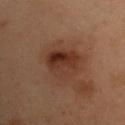site: the right upper arm | patient: male, aged approximately 55 | image source: total-body-photography crop, ~15 mm field of view | TBP lesion metrics: an area of roughly 13 mm², an outline eccentricity of about 0.55 (0 = round, 1 = elongated), and a symmetry-axis asymmetry near 0.15; a lesion color around L≈26 a*≈18 b*≈23 in CIELAB, roughly 7 lightness units darker than nearby skin, and a lesion-to-skin contrast of about 8 (normalized; higher = more distinct) | tile lighting: cross-polarized illumination | lesion diameter: about 4.5 mm.